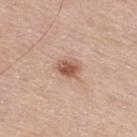No biopsy was performed on this lesion — it was imaged during a full skin examination and was not determined to be concerning.
From the upper back.
A close-up tile cropped from a whole-body skin photograph, about 15 mm across.
About 3 mm across.
This is a white-light tile.
The lesion-visualizer software estimated a border-irregularity index near 2/10. The analysis additionally found an automated nevus-likeness rating near 90 out of 100 and a lesion-detection confidence of about 100/100.
The subject is a male in their 80s.